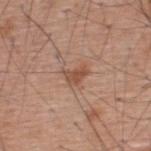follow-up = catalogued during a skin exam; not biopsied | lesion size = ~2.5 mm (longest diameter) | image = total-body-photography crop, ~15 mm field of view | subject = male, aged approximately 60 | lighting = white-light illumination | anatomic site = the back.